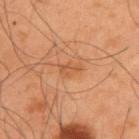Q: Is there a histopathology result?
A: no biopsy performed (imaged during a skin exam)
Q: What are the patient's age and sex?
A: male, aged 53–57
Q: What did automated image analysis measure?
A: a border-irregularity rating of about 4.5/10, a within-lesion color-variation index near 0.5/10, and radial color variation of about 0; a nevus-likeness score of about 30/100 and a detector confidence of about 100 out of 100 that the crop contains a lesion
Q: What kind of image is this?
A: ~15 mm tile from a whole-body skin photo
Q: Illumination type?
A: cross-polarized
Q: What is the lesion's diameter?
A: ~2.5 mm (longest diameter)
Q: Lesion location?
A: the upper back